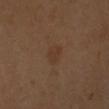{"site": "left forearm", "lesion_size": {"long_diameter_mm_approx": 2.5}, "lighting": "cross-polarized", "automated_metrics": {"area_mm2_approx": 4.0, "eccentricity": 0.7, "shape_asymmetry": 0.3, "cielab_L": 35, "cielab_a": 17, "cielab_b": 28, "vs_skin_darker_L": 5.0}, "patient": {"sex": "female", "age_approx": 55}, "image": {"source": "total-body photography crop", "field_of_view_mm": 15}}